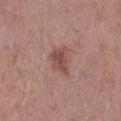An algorithmic analysis of the crop reported a mean CIELAB color near L≈49 a*≈22 b*≈24 and about 9 CIELAB-L* units darker than the surrounding skin. A lesion tile, about 15 mm wide, cut from a 3D total-body photograph. A male subject, aged 48 to 52. Located on the right lower leg.